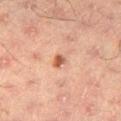Findings:
- notes: total-body-photography surveillance lesion; no biopsy
- automated lesion analysis: border irregularity of about 2.5 on a 0–10 scale, a within-lesion color-variation index near 4.5/10, and radial color variation of about 1; an automated nevus-likeness rating near 85 out of 100 and lesion-presence confidence of about 100/100
- imaging modality: ~15 mm crop, total-body skin-cancer survey
- lighting: cross-polarized illumination
- subject: male, approximately 50 years of age
- anatomic site: the leg
- diameter: ≈2.5 mm